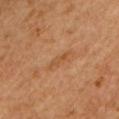No biopsy was performed on this lesion — it was imaged during a full skin examination and was not determined to be concerning.
The lesion's longest dimension is about 3 mm.
A female subject aged 63 to 67.
On the chest.
Cropped from a whole-body photographic skin survey; the tile spans about 15 mm.